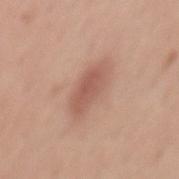{"biopsy_status": "not biopsied; imaged during a skin examination", "automated_metrics": {"border_irregularity_0_10": 3.0, "color_variation_0_10": 2.5, "nevus_likeness_0_100": 75, "lesion_detection_confidence_0_100": 100}, "image": {"source": "total-body photography crop", "field_of_view_mm": 15}, "site": "abdomen", "patient": {"sex": "female", "age_approx": 35}}